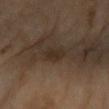About 2.5 mm across. The patient is a female aged 68–72. The lesion is on the right forearm. The total-body-photography lesion software estimated a footprint of about 3.5 mm², a shape eccentricity near 0.75, and two-axis asymmetry of about 0.25. The analysis additionally found border irregularity of about 2.5 on a 0–10 scale and peripheral color asymmetry of about 0.5. Captured under cross-polarized illumination. A 15 mm crop from a total-body photograph taken for skin-cancer surveillance.